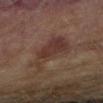Impression: The lesion was tiled from a total-body skin photograph and was not biopsied. Acquisition and patient details: On the right forearm. The subject is a female about 60 years old. A lesion tile, about 15 mm wide, cut from a 3D total-body photograph.